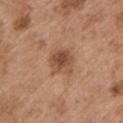notes — catalogued during a skin exam; not biopsied
image source — 15 mm crop, total-body photography
lesion size — about 3.5 mm
location — the left upper arm
patient — male, about 55 years old